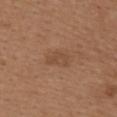biopsy status: total-body-photography surveillance lesion; no biopsy | lighting: white-light | lesion size: ≈3 mm | image source: total-body-photography crop, ~15 mm field of view | anatomic site: the upper back | subject: female, aged around 40.